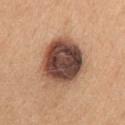Part of a total-body skin-imaging series; this lesion was reviewed on a skin check and was not flagged for biopsy. Located on the right upper arm. Cropped from a total-body skin-imaging series; the visible field is about 15 mm. A female subject in their mid-40s. The total-body-photography lesion software estimated a mean CIELAB color near L≈46 a*≈20 b*≈27, roughly 21 lightness units darker than nearby skin, and a normalized lesion–skin contrast near 14.5. It also reported a border-irregularity index near 1.5/10 and a color-variation rating of about 9/10. Longest diameter approximately 6 mm. This is a white-light tile.